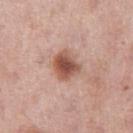Case summary:
* workup — total-body-photography surveillance lesion; no biopsy
* lesion diameter — about 3.5 mm
* image source — 15 mm crop, total-body photography
* anatomic site — the right thigh
* tile lighting — white-light illumination
* patient — female, about 50 years old
* TBP lesion metrics — a footprint of about 8 mm², an outline eccentricity of about 0.5 (0 = round, 1 = elongated), and a symmetry-axis asymmetry near 0.25; a border-irregularity index near 2.5/10 and a color-variation rating of about 5/10